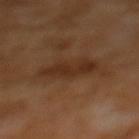Acquisition and patient details:
Approximately 5.5 mm at its widest. On the back. A region of skin cropped from a whole-body photographic capture, roughly 15 mm wide. A female patient in their mid-50s.